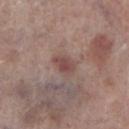Clinical impression:
The lesion was tiled from a total-body skin photograph and was not biopsied.
Background:
A male patient roughly 70 years of age. Cropped from a whole-body photographic skin survey; the tile spans about 15 mm. Imaged with white-light lighting. The recorded lesion diameter is about 2.5 mm. From the right lower leg.